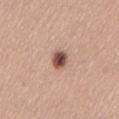notes: no biopsy performed (imaged during a skin exam)
patient: female, aged approximately 30
body site: the lower back
size: ~3 mm (longest diameter)
automated metrics: an outline eccentricity of about 0.75 (0 = round, 1 = elongated) and a shape-asymmetry score of about 0.25 (0 = symmetric); a mean CIELAB color near L≈50 a*≈20 b*≈24, a lesion–skin lightness drop of about 18, and a normalized border contrast of about 12; a border-irregularity rating of about 2/10, internal color variation of about 6 on a 0–10 scale, and a peripheral color-asymmetry measure near 2
lighting: white-light illumination
image: ~15 mm tile from a whole-body skin photo